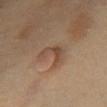automated lesion analysis: a footprint of about 6.5 mm² and a shape-asymmetry score of about 0.65 (0 = symmetric); location: the front of the torso; acquisition: ~15 mm crop, total-body skin-cancer survey; lighting: cross-polarized illumination; patient: female, approximately 55 years of age; size: ~4 mm (longest diameter).